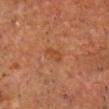subject=male, in their mid- to late 60s | lesion diameter=≈2.5 mm | image=~15 mm tile from a whole-body skin photo | lighting=cross-polarized | site=the head or neck | automated lesion analysis=a lesion color around L≈44 a*≈25 b*≈36 in CIELAB and a normalized lesion–skin contrast near 6; an automated nevus-likeness rating near 0 out of 100.